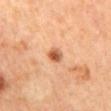Q: Was a biopsy performed?
A: total-body-photography surveillance lesion; no biopsy
Q: Lesion location?
A: the mid back
Q: What are the patient's age and sex?
A: female, aged around 65
Q: How was the tile lit?
A: cross-polarized illumination
Q: Automated lesion metrics?
A: a lesion color around L≈57 a*≈26 b*≈37 in CIELAB and a normalized border contrast of about 9; a border-irregularity rating of about 2/10 and a peripheral color-asymmetry measure near 2; an automated nevus-likeness rating near 95 out of 100 and a lesion-detection confidence of about 100/100
Q: What is the imaging modality?
A: ~15 mm crop, total-body skin-cancer survey